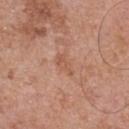Imaged during a routine full-body skin examination; the lesion was not biopsied and no histopathology is available.
A 15 mm close-up extracted from a 3D total-body photography capture.
Located on the chest.
Measured at roughly 3 mm in maximum diameter.
The patient is a male in their 70s.
Imaged with white-light lighting.
An algorithmic analysis of the crop reported an average lesion color of about L≈55 a*≈23 b*≈31 (CIELAB), a lesion–skin lightness drop of about 7, and a lesion-to-skin contrast of about 5 (normalized; higher = more distinct).